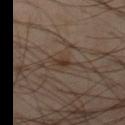Impression: Recorded during total-body skin imaging; not selected for excision or biopsy. Background: This image is a 15 mm lesion crop taken from a total-body photograph. Imaged with cross-polarized lighting. Automated tile analysis of the lesion measured a footprint of about 2.5 mm², an outline eccentricity of about 0.9 (0 = round, 1 = elongated), and a symmetry-axis asymmetry near 0.3. And it measured a nevus-likeness score of about 90/100 and lesion-presence confidence of about 95/100. The recorded lesion diameter is about 3 mm. The lesion is located on the left thigh. A male subject, aged 48 to 52.